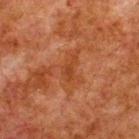Part of a total-body skin-imaging series; this lesion was reviewed on a skin check and was not flagged for biopsy. Automated image analysis of the tile measured a lesion color around L≈34 a*≈24 b*≈32 in CIELAB, roughly 5 lightness units darker than nearby skin, and a normalized border contrast of about 5.5. The analysis additionally found a nevus-likeness score of about 0/100 and lesion-presence confidence of about 100/100. Imaged with cross-polarized lighting. The patient is a male aged 78–82. A close-up tile cropped from a whole-body skin photograph, about 15 mm across. Located on the upper back.